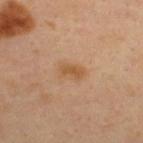{
  "biopsy_status": "not biopsied; imaged during a skin examination",
  "image": {
    "source": "total-body photography crop",
    "field_of_view_mm": 15
  },
  "site": "upper back",
  "automated_metrics": {
    "cielab_L": 52,
    "cielab_a": 19,
    "cielab_b": 35,
    "vs_skin_darker_L": 7.0,
    "lesion_detection_confidence_0_100": 100
  },
  "lesion_size": {
    "long_diameter_mm_approx": 3.0
  },
  "lighting": "cross-polarized",
  "patient": {
    "sex": "male",
    "age_approx": 35
  }
}